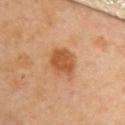Captured during whole-body skin photography for melanoma surveillance; the lesion was not biopsied. Located on the upper back. A female patient, aged 58–62. Imaged with cross-polarized lighting. A roughly 15 mm field-of-view crop from a total-body skin photograph.